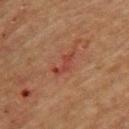Recorded during total-body skin imaging; not selected for excision or biopsy.
From the upper back.
Approximately 3 mm at its widest.
This is a cross-polarized tile.
A 15 mm close-up tile from a total-body photography series done for melanoma screening.
A female patient, about 70 years old.